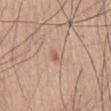Impression: Part of a total-body skin-imaging series; this lesion was reviewed on a skin check and was not flagged for biopsy. Background: The lesion is located on the right thigh. A male subject, about 60 years old. Cropped from a whole-body photographic skin survey; the tile spans about 15 mm. The total-body-photography lesion software estimated a lesion area of about 1 mm² and a shape eccentricity near 0.7. It also reported a border-irregularity rating of about 3/10, a within-lesion color-variation index near 0/10, and radial color variation of about 0. The software also gave a detector confidence of about 100 out of 100 that the crop contains a lesion. The recorded lesion diameter is about 1 mm.